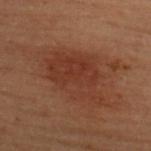{
  "biopsy_status": "not biopsied; imaged during a skin examination"
}